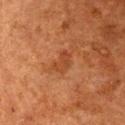notes = total-body-photography surveillance lesion; no biopsy
image = total-body-photography crop, ~15 mm field of view
location = the front of the torso
patient = male, approximately 80 years of age
tile lighting = cross-polarized illumination
image-analysis metrics = border irregularity of about 5 on a 0–10 scale and a peripheral color-asymmetry measure near 0.5; an automated nevus-likeness rating near 0 out of 100 and a detector confidence of about 100 out of 100 that the crop contains a lesion